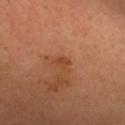No biopsy was performed on this lesion — it was imaged during a full skin examination and was not determined to be concerning.
A 15 mm close-up extracted from a 3D total-body photography capture.
The subject is a female roughly 45 years of age.
The lesion's longest dimension is about 2 mm.
On the head or neck.
The tile uses cross-polarized illumination.